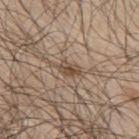Q: Was a biopsy performed?
A: total-body-photography surveillance lesion; no biopsy
Q: What are the patient's age and sex?
A: male, in their 70s
Q: What lighting was used for the tile?
A: white-light
Q: What is the imaging modality?
A: ~15 mm tile from a whole-body skin photo
Q: Where on the body is the lesion?
A: the mid back
Q: What is the lesion's diameter?
A: ≈3 mm